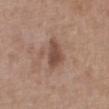Impression:
Part of a total-body skin-imaging series; this lesion was reviewed on a skin check and was not flagged for biopsy.
Acquisition and patient details:
Captured under white-light illumination. Measured at roughly 4 mm in maximum diameter. Automated tile analysis of the lesion measured a lesion color around L≈48 a*≈19 b*≈26 in CIELAB, roughly 11 lightness units darker than nearby skin, and a normalized border contrast of about 8. The software also gave a detector confidence of about 100 out of 100 that the crop contains a lesion. The lesion is located on the chest. A region of skin cropped from a whole-body photographic capture, roughly 15 mm wide. A female patient roughly 75 years of age.